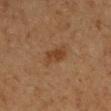Case summary:
• workup · no biopsy performed (imaged during a skin exam)
• site · the left forearm
• tile lighting · cross-polarized illumination
• image source · total-body-photography crop, ~15 mm field of view
• patient · female, aged 38–42
• automated metrics · an area of roughly 5 mm², an eccentricity of roughly 0.9, and two-axis asymmetry of about 0.3; a color-variation rating of about 1.5/10 and peripheral color asymmetry of about 0.5; an automated nevus-likeness rating near 70 out of 100 and a lesion-detection confidence of about 100/100
• size · ~3.5 mm (longest diameter)